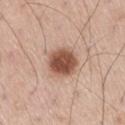| feature | finding |
|---|---|
| workup | no biopsy performed (imaged during a skin exam) |
| illumination | white-light illumination |
| lesion size | ≈4 mm |
| patient | male, in their 60s |
| site | the right thigh |
| image source | ~15 mm tile from a whole-body skin photo |